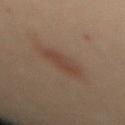This lesion was catalogued during total-body skin photography and was not selected for biopsy.
A region of skin cropped from a whole-body photographic capture, roughly 15 mm wide.
The lesion is on the mid back.
The recorded lesion diameter is about 4 mm.
Captured under cross-polarized illumination.
The patient is a female aged approximately 30.